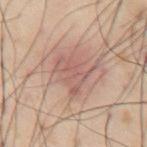Q: Illumination type?
A: cross-polarized illumination
Q: Lesion location?
A: the abdomen
Q: What are the patient's age and sex?
A: male, about 55 years old
Q: How was this image acquired?
A: ~15 mm tile from a whole-body skin photo
Q: What did automated image analysis measure?
A: an area of roughly 10 mm², an eccentricity of roughly 0.55, and a symmetry-axis asymmetry near 0.5